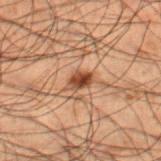Findings:
* follow-up: catalogued during a skin exam; not biopsied
* patient: male, about 50 years old
* tile lighting: cross-polarized illumination
* automated metrics: a footprint of about 3.5 mm², an eccentricity of roughly 0.7, and a symmetry-axis asymmetry near 0.25; a mean CIELAB color near L≈35 a*≈20 b*≈28, a lesion–skin lightness drop of about 13, and a lesion-to-skin contrast of about 11 (normalized; higher = more distinct); an automated nevus-likeness rating near 95 out of 100
* imaging modality: ~15 mm tile from a whole-body skin photo
* anatomic site: the right lower leg
* lesion size: ≈2.5 mm